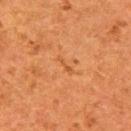<lesion>
  <biopsy_status>not biopsied; imaged during a skin examination</biopsy_status>
  <image>
    <source>total-body photography crop</source>
    <field_of_view_mm>15</field_of_view_mm>
  </image>
  <patient>
    <sex>female</sex>
    <age_approx>50</age_approx>
  </patient>
  <site>right upper arm</site>
</lesion>